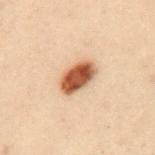Notes:
- notes · no biopsy performed (imaged during a skin exam)
- tile lighting · cross-polarized
- TBP lesion metrics · a footprint of about 9.5 mm² and a symmetry-axis asymmetry near 0.15; a lesion color around L≈46 a*≈20 b*≈30 in CIELAB, about 17 CIELAB-L* units darker than the surrounding skin, and a normalized border contrast of about 12.5
- image source · ~15 mm crop, total-body skin-cancer survey
- patient · female, aged around 30
- site · the mid back